Longest diameter approximately 7 mm · The total-body-photography lesion software estimated a detector confidence of about 95 out of 100 that the crop contains a lesion · on the chest · cropped from a total-body skin-imaging series; the visible field is about 15 mm · the patient is a male aged 68 to 72 — 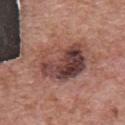Conclusion:
Histopathology of the biopsied lesion showed an invasive melanoma arising in association with a nevus (Breslow thickness 0.4 mm, mitotic rate <1/mm²).